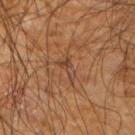No biopsy was performed on this lesion — it was imaged during a full skin examination and was not determined to be concerning. The patient is a male in their 60s. Imaged with cross-polarized lighting. A 15 mm close-up tile from a total-body photography series done for melanoma screening. From the right arm.